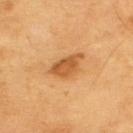<case>
<biopsy_status>not biopsied; imaged during a skin examination</biopsy_status>
<image>
  <source>total-body photography crop</source>
  <field_of_view_mm>15</field_of_view_mm>
</image>
<lighting>cross-polarized</lighting>
<lesion_size>
  <long_diameter_mm_approx>4.5</long_diameter_mm_approx>
</lesion_size>
<automated_metrics>
  <area_mm2_approx>7.5</area_mm2_approx>
  <eccentricity>0.8</eccentricity>
  <shape_asymmetry>0.3</shape_asymmetry>
</automated_metrics>
<patient>
  <sex>male</sex>
  <age_approx>60</age_approx>
</patient>
<site>upper back</site>
</case>